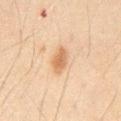Case summary:
• notes · imaged on a skin check; not biopsied
• lesion size · ~3 mm (longest diameter)
• image · ~15 mm crop, total-body skin-cancer survey
• patient · male, approximately 65 years of age
• tile lighting · cross-polarized illumination
• location · the abdomen